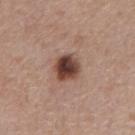Imaged during a routine full-body skin examination; the lesion was not biopsied and no histopathology is available. This image is a 15 mm lesion crop taken from a total-body photograph. A male subject, aged approximately 55. The tile uses white-light illumination. Approximately 3 mm at its widest.